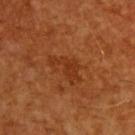| field | value |
|---|---|
| follow-up | catalogued during a skin exam; not biopsied |
| subject | male, aged 58 to 62 |
| lesion diameter | ~4 mm (longest diameter) |
| illumination | cross-polarized illumination |
| automated metrics | a lesion color around L≈32 a*≈25 b*≈35 in CIELAB, a lesion–skin lightness drop of about 6, and a normalized border contrast of about 6; a border-irregularity rating of about 8/10 and peripheral color asymmetry of about 0.5; a nevus-likeness score of about 0/100 and lesion-presence confidence of about 100/100 |
| acquisition | ~15 mm tile from a whole-body skin photo |
| site | the upper back |